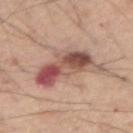diameter: ~7 mm (longest diameter) | tile lighting: white-light illumination | image source: ~15 mm crop, total-body skin-cancer survey | location: the arm | subject: male, aged approximately 60.